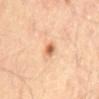Q: Was this lesion biopsied?
A: catalogued during a skin exam; not biopsied
Q: Patient demographics?
A: male, approximately 55 years of age
Q: What is the lesion's diameter?
A: ~3.5 mm (longest diameter)
Q: What is the imaging modality?
A: ~15 mm crop, total-body skin-cancer survey
Q: Where on the body is the lesion?
A: the mid back
Q: What lighting was used for the tile?
A: cross-polarized